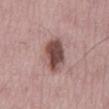Imaged during a routine full-body skin examination; the lesion was not biopsied and no histopathology is available. A roughly 15 mm field-of-view crop from a total-body skin photograph. The recorded lesion diameter is about 6 mm. This is a white-light tile. From the mid back. The patient is a male aged 68 to 72.